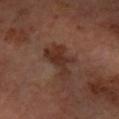{"biopsy_status": "not biopsied; imaged during a skin examination"}